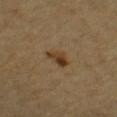Assessment: Captured during whole-body skin photography for melanoma surveillance; the lesion was not biopsied.